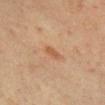Assessment: No biopsy was performed on this lesion — it was imaged during a full skin examination and was not determined to be concerning. Acquisition and patient details: This is a cross-polarized tile. Measured at roughly 2.5 mm in maximum diameter. On the chest. A female patient, aged around 40. A 15 mm close-up tile from a total-body photography series done for melanoma screening.